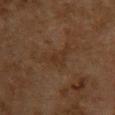  biopsy_status: not biopsied; imaged during a skin examination
  site: upper back
  image:
    source: total-body photography crop
    field_of_view_mm: 15
  patient:
    sex: female
    age_approx: 60